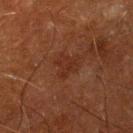  biopsy_status: not biopsied; imaged during a skin examination
  lighting: cross-polarized
  site: right lower leg
  image:
    source: total-body photography crop
    field_of_view_mm: 15
  patient:
    sex: male
    age_approx: 65